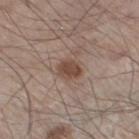Q: Is there a histopathology result?
A: no biopsy performed (imaged during a skin exam)
Q: How was this image acquired?
A: ~15 mm tile from a whole-body skin photo
Q: Where on the body is the lesion?
A: the left lower leg
Q: Automated lesion metrics?
A: an area of roughly 5 mm², an eccentricity of roughly 0.6, and two-axis asymmetry of about 0.15; a mean CIELAB color near L≈46 a*≈17 b*≈25, roughly 10 lightness units darker than nearby skin, and a normalized lesion–skin contrast near 8.5
Q: Lesion size?
A: ≈3 mm
Q: Patient demographics?
A: male, aged 58 to 62